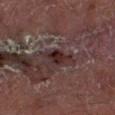Approximately 3 mm at its widest. Captured under cross-polarized illumination. The patient is a male aged 53 to 57. The lesion is located on the right lower leg. A 15 mm close-up tile from a total-body photography series done for melanoma screening.